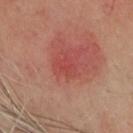Acquisition and patient details:
A female subject roughly 55 years of age. About 2.5 mm across. A lesion tile, about 15 mm wide, cut from a 3D total-body photograph. An algorithmic analysis of the crop reported a lesion color around L≈48 a*≈35 b*≈28 in CIELAB, roughly 5 lightness units darker than nearby skin, and a normalized lesion–skin contrast near 4. And it measured a border-irregularity rating of about 3/10, internal color variation of about 2 on a 0–10 scale, and radial color variation of about 0.5. And it measured an automated nevus-likeness rating near 0 out of 100 and a detector confidence of about 100 out of 100 that the crop contains a lesion. The lesion is on the head or neck. Captured under cross-polarized illumination.